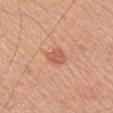Impression:
Captured during whole-body skin photography for melanoma surveillance; the lesion was not biopsied.
Clinical summary:
The lesion is on the chest. This is a white-light tile. A female subject about 45 years old. Cropped from a whole-body photographic skin survey; the tile spans about 15 mm. The total-body-photography lesion software estimated a lesion area of about 4 mm² and a shape eccentricity near 0.7. The software also gave a mean CIELAB color near L≈58 a*≈29 b*≈33, a lesion–skin lightness drop of about 10, and a normalized border contrast of about 6.5. About 2.5 mm across.